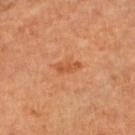Findings:
• notes: imaged on a skin check; not biopsied
• anatomic site: the left lower leg
• image: total-body-photography crop, ~15 mm field of view
• subject: aged 68 to 72
• size: ≈2.5 mm
• image-analysis metrics: a lesion color around L≈53 a*≈29 b*≈40 in CIELAB, roughly 8 lightness units darker than nearby skin, and a lesion-to-skin contrast of about 6.5 (normalized; higher = more distinct); border irregularity of about 3.5 on a 0–10 scale, a within-lesion color-variation index near 0/10, and a peripheral color-asymmetry measure near 0
• illumination: cross-polarized